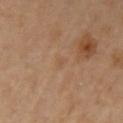The total-body-photography lesion software estimated a footprint of about 2 mm², an eccentricity of roughly 0.65, and two-axis asymmetry of about 0.35. And it measured a normalized border contrast of about 3.5. And it measured a border-irregularity index near 3.5/10, a color-variation rating of about 0.5/10, and a peripheral color-asymmetry measure near 0. It also reported an automated nevus-likeness rating near 0 out of 100 and a detector confidence of about 100 out of 100 that the crop contains a lesion. The lesion is located on the left upper arm. Cropped from a whole-body photographic skin survey; the tile spans about 15 mm. Longest diameter approximately 2 mm. A female patient aged 63 to 67. This is a cross-polarized tile.